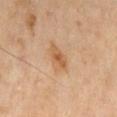biopsy status: imaged on a skin check; not biopsied | patient: male, aged 68 to 72 | anatomic site: the lower back | image: ~15 mm tile from a whole-body skin photo.